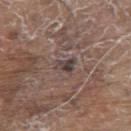biopsy status: imaged on a skin check; not biopsied | patient: male, roughly 80 years of age | site: the upper back | image source: ~15 mm crop, total-body skin-cancer survey.